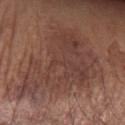notes — catalogued during a skin exam; not biopsied
size — ~11.5 mm (longest diameter)
anatomic site — the right forearm
image source — ~15 mm crop, total-body skin-cancer survey
lighting — white-light
subject — male, aged 68–72